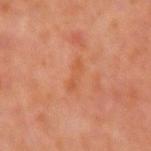illumination: cross-polarized illumination | lesion diameter: ~3.5 mm (longest diameter) | image source: total-body-photography crop, ~15 mm field of view | subject: female, approximately 50 years of age | site: the left forearm | TBP lesion metrics: a lesion-detection confidence of about 100/100.